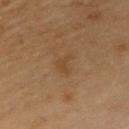{"biopsy_status": "not biopsied; imaged during a skin examination", "patient": {"sex": "male", "age_approx": 55}, "site": "chest", "image": {"source": "total-body photography crop", "field_of_view_mm": 15}, "lighting": "cross-polarized", "lesion_size": {"long_diameter_mm_approx": 2.5}}